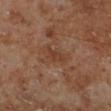- biopsy status · no biopsy performed (imaged during a skin exam)
- patient · male, about 70 years old
- automated metrics · an average lesion color of about L≈37 a*≈21 b*≈29 (CIELAB), a lesion–skin lightness drop of about 6, and a lesion-to-skin contrast of about 6 (normalized; higher = more distinct); a nevus-likeness score of about 0/100 and a lesion-detection confidence of about 100/100
- illumination · cross-polarized
- size · ~3 mm (longest diameter)
- imaging modality · ~15 mm crop, total-body skin-cancer survey
- location · the left lower leg